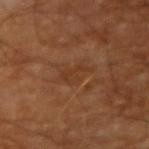Part of a total-body skin-imaging series; this lesion was reviewed on a skin check and was not flagged for biopsy. A 15 mm close-up extracted from a 3D total-body photography capture. A male subject, about 60 years old. On the right upper arm. The lesion's longest dimension is about 3.5 mm.